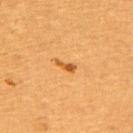Assessment: The lesion was photographed on a routine skin check and not biopsied; there is no pathology result. Background: Located on the upper back. This is a cross-polarized tile. A female subject aged approximately 55. A region of skin cropped from a whole-body photographic capture, roughly 15 mm wide. Measured at roughly 2.5 mm in maximum diameter.